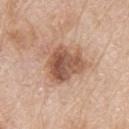| feature | finding |
|---|---|
| workup | no biopsy performed (imaged during a skin exam) |
| image-analysis metrics | a border-irregularity rating of about 3.5/10, a color-variation rating of about 6.5/10, and radial color variation of about 2.5 |
| subject | male, approximately 80 years of age |
| lesion diameter | about 4.5 mm |
| image | total-body-photography crop, ~15 mm field of view |
| body site | the upper back |